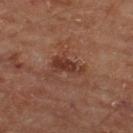Notes:
• TBP lesion metrics — a lesion color around L≈35 a*≈22 b*≈27 in CIELAB, about 9 CIELAB-L* units darker than the surrounding skin, and a normalized border contrast of about 7.5; a nevus-likeness score of about 0/100 and a detector confidence of about 100 out of 100 that the crop contains a lesion
• lighting — cross-polarized illumination
• image source — total-body-photography crop, ~15 mm field of view
• lesion size — ~3.5 mm (longest diameter)
• patient — male, in their mid-60s
• body site — the back